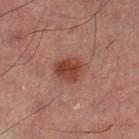image source — 15 mm crop, total-body photography | lesion diameter — ~3.5 mm (longest diameter) | site — the left thigh | tile lighting — cross-polarized.